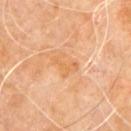Assessment:
Recorded during total-body skin imaging; not selected for excision or biopsy.
Image and clinical context:
A region of skin cropped from a whole-body photographic capture, roughly 15 mm wide. From the upper back. A male patient in their 60s. Measured at roughly 3 mm in maximum diameter. Automated tile analysis of the lesion measured an area of roughly 4.5 mm² and two-axis asymmetry of about 0.6. The analysis additionally found a lesion-detection confidence of about 100/100. The tile uses cross-polarized illumination.